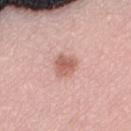Imaged during a routine full-body skin examination; the lesion was not biopsied and no histopathology is available.
On the lower back.
The subject is a female approximately 40 years of age.
A close-up tile cropped from a whole-body skin photograph, about 15 mm across.
Captured under white-light illumination.